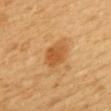{"biopsy_status": "not biopsied; imaged during a skin examination", "patient": {"sex": "female", "age_approx": 55}, "lesion_size": {"long_diameter_mm_approx": 3.0}, "automated_metrics": {"eccentricity": 0.55, "shape_asymmetry": 0.2, "cielab_L": 56, "cielab_a": 25, "cielab_b": 45, "vs_skin_darker_L": 10.0, "vs_skin_contrast_norm": 7.0}, "image": {"source": "total-body photography crop", "field_of_view_mm": 15}, "site": "mid back"}